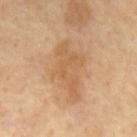Impression:
Part of a total-body skin-imaging series; this lesion was reviewed on a skin check and was not flagged for biopsy.
Context:
Located on the mid back. A male subject roughly 70 years of age. A 15 mm close-up tile from a total-body photography series done for melanoma screening.